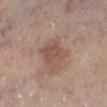Imaged during a routine full-body skin examination; the lesion was not biopsied and no histopathology is available.
A female subject, aged around 65.
A 15 mm close-up extracted from a 3D total-body photography capture.
On the right lower leg.